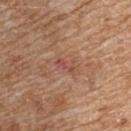<tbp_lesion>
  <image>
    <source>total-body photography crop</source>
    <field_of_view_mm>15</field_of_view_mm>
  </image>
  <site>upper back</site>
  <patient>
    <sex>female</sex>
    <age_approx>70</age_approx>
  </patient>
</tbp_lesion>